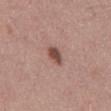Impression:
No biopsy was performed on this lesion — it was imaged during a full skin examination and was not determined to be concerning.
Acquisition and patient details:
A 15 mm crop from a total-body photograph taken for skin-cancer surveillance. A male subject, roughly 45 years of age. The lesion is on the abdomen.